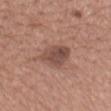No biopsy was performed on this lesion — it was imaged during a full skin examination and was not determined to be concerning. The tile uses white-light illumination. The patient is a female aged 53–57. A close-up tile cropped from a whole-body skin photograph, about 15 mm across. Located on the mid back. About 4 mm across.